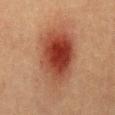| field | value |
|---|---|
| biopsy status | imaged on a skin check; not biopsied |
| image source | total-body-photography crop, ~15 mm field of view |
| site | the abdomen |
| patient | male, aged approximately 40 |